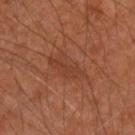Clinical impression: The lesion was photographed on a routine skin check and not biopsied; there is no pathology result. Clinical summary: A roughly 15 mm field-of-view crop from a total-body skin photograph. The lesion is on the arm. A male subject aged around 45.